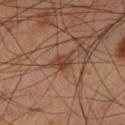{"biopsy_status": "not biopsied; imaged during a skin examination", "patient": {"sex": "male", "age_approx": 60}, "site": "left lower leg", "automated_metrics": {"area_mm2_approx": 4.0, "eccentricity": 0.75, "shape_asymmetry": 0.3, "cielab_L": 40, "cielab_a": 21, "cielab_b": 30, "vs_skin_darker_L": 9.0, "vs_skin_contrast_norm": 7.5}, "lighting": "cross-polarized", "image": {"source": "total-body photography crop", "field_of_view_mm": 15}, "lesion_size": {"long_diameter_mm_approx": 2.5}}